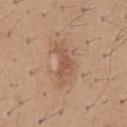The lesion was tiled from a total-body skin photograph and was not biopsied.
Imaged with white-light lighting.
A male subject, in their 40s.
The lesion is located on the mid back.
A roughly 15 mm field-of-view crop from a total-body skin photograph.
Measured at roughly 5 mm in maximum diameter.